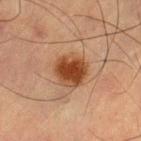Captured during whole-body skin photography for melanoma surveillance; the lesion was not biopsied. On the right thigh. The patient is a male in their mid-60s. The recorded lesion diameter is about 4 mm. A region of skin cropped from a whole-body photographic capture, roughly 15 mm wide.